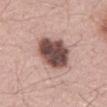Notes:
* follow-up — imaged on a skin check; not biopsied
* patient — male, approximately 60 years of age
* lesion diameter — about 5 mm
* location — the abdomen
* imaging modality — ~15 mm crop, total-body skin-cancer survey